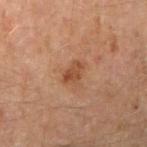Recorded during total-body skin imaging; not selected for excision or biopsy. The tile uses cross-polarized illumination. Measured at roughly 2.5 mm in maximum diameter. This image is a 15 mm lesion crop taken from a total-body photograph. A male subject in their mid-40s. From the right upper arm. The lesion-visualizer software estimated a lesion color around L≈41 a*≈20 b*≈30 in CIELAB and roughly 8 lightness units darker than nearby skin. The analysis additionally found a border-irregularity index near 3/10, a within-lesion color-variation index near 3.5/10, and a peripheral color-asymmetry measure near 1.5. It also reported a nevus-likeness score of about 10/100 and a lesion-detection confidence of about 100/100.